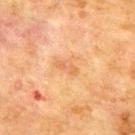notes = total-body-photography surveillance lesion; no biopsy | acquisition = ~15 mm crop, total-body skin-cancer survey | subject = male, aged 68 to 72 | site = the upper back.